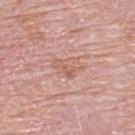workup=no biopsy performed (imaged during a skin exam)
patient=female, aged approximately 65
diameter=~3.5 mm (longest diameter)
imaging modality=total-body-photography crop, ~15 mm field of view
site=the upper back
automated lesion analysis=a nevus-likeness score of about 0/100 and a detector confidence of about 100 out of 100 that the crop contains a lesion
tile lighting=white-light illumination